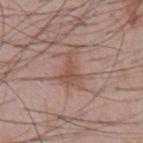The lesion was tiled from a total-body skin photograph and was not biopsied.